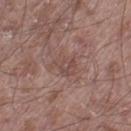Case summary:
- notes — total-body-photography surveillance lesion; no biopsy
- location — the left thigh
- image — total-body-photography crop, ~15 mm field of view
- diameter — ~3.5 mm (longest diameter)
- patient — male, aged around 55